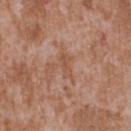Q: Was this lesion biopsied?
A: catalogued during a skin exam; not biopsied
Q: What are the patient's age and sex?
A: male, aged 43–47
Q: Lesion location?
A: the upper back
Q: What did automated image analysis measure?
A: a shape-asymmetry score of about 0.35 (0 = symmetric); a color-variation rating of about 1/10 and peripheral color asymmetry of about 0.5
Q: What lighting was used for the tile?
A: white-light
Q: What kind of image is this?
A: total-body-photography crop, ~15 mm field of view
Q: Lesion size?
A: ~3 mm (longest diameter)